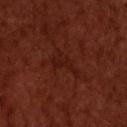biopsy status — no biopsy performed (imaged during a skin exam)
tile lighting — cross-polarized
location — the upper back
subject — male, aged approximately 50
imaging modality — ~15 mm tile from a whole-body skin photo
image-analysis metrics — a mean CIELAB color near L≈19 a*≈25 b*≈24, about 4 CIELAB-L* units darker than the surrounding skin, and a lesion-to-skin contrast of about 5 (normalized; higher = more distinct); a within-lesion color-variation index near 0.5/10 and radial color variation of about 0
diameter — ~3.5 mm (longest diameter)